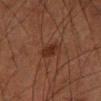Impression: Part of a total-body skin-imaging series; this lesion was reviewed on a skin check and was not flagged for biopsy. Acquisition and patient details: The lesion is located on the left thigh. Automated tile analysis of the lesion measured a classifier nevus-likeness of about 85/100. The lesion's longest dimension is about 2.5 mm. The subject is a male aged approximately 80. A 15 mm close-up tile from a total-body photography series done for melanoma screening. Captured under cross-polarized illumination.